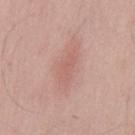Q: Was this lesion biopsied?
A: catalogued during a skin exam; not biopsied
Q: What is the lesion's diameter?
A: ~3.5 mm (longest diameter)
Q: Automated lesion metrics?
A: a lesion area of about 5 mm²; a border-irregularity rating of about 3.5/10, internal color variation of about 1 on a 0–10 scale, and peripheral color asymmetry of about 0.5
Q: Lesion location?
A: the mid back
Q: What are the patient's age and sex?
A: male, approximately 40 years of age
Q: What kind of image is this?
A: 15 mm crop, total-body photography
Q: What lighting was used for the tile?
A: white-light